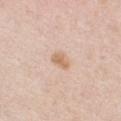Captured during whole-body skin photography for melanoma surveillance; the lesion was not biopsied. A female patient, in their 40s. Captured under white-light illumination. The lesion is on the chest. Cropped from a total-body skin-imaging series; the visible field is about 15 mm. Longest diameter approximately 2.5 mm.